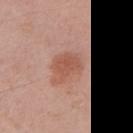biopsy status: catalogued during a skin exam; not biopsied | diameter: ≈4 mm | body site: the chest | patient: male, aged around 55 | imaging modality: total-body-photography crop, ~15 mm field of view | lighting: white-light | image-analysis metrics: a footprint of about 8.5 mm², an outline eccentricity of about 0.7 (0 = round, 1 = elongated), and a shape-asymmetry score of about 0.3 (0 = symmetric); a border-irregularity index near 3.5/10 and a peripheral color-asymmetry measure near 1; a nevus-likeness score of about 85/100 and a detector confidence of about 100 out of 100 that the crop contains a lesion.